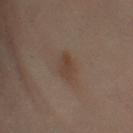Captured during whole-body skin photography for melanoma surveillance; the lesion was not biopsied. The lesion is located on the leg. The lesion-visualizer software estimated a lesion area of about 5 mm², a shape eccentricity near 0.75, and two-axis asymmetry of about 0.3. It also reported border irregularity of about 2.5 on a 0–10 scale, internal color variation of about 2 on a 0–10 scale, and radial color variation of about 0.5. And it measured a classifier nevus-likeness of about 35/100 and a detector confidence of about 100 out of 100 that the crop contains a lesion. Cropped from a whole-body photographic skin survey; the tile spans about 15 mm. The recorded lesion diameter is about 3 mm. A female subject about 60 years old. This is a cross-polarized tile.